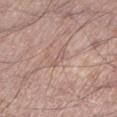follow-up = no biopsy performed (imaged during a skin exam); lesion size = ≈3 mm; site = the left lower leg; imaging modality = 15 mm crop, total-body photography; subject = male, about 60 years old; illumination = white-light.